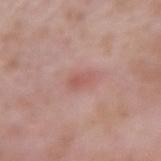Q: Is there a histopathology result?
A: catalogued during a skin exam; not biopsied
Q: Who is the patient?
A: male, about 55 years old
Q: What is the lesion's diameter?
A: about 2.5 mm
Q: Automated lesion metrics?
A: an area of roughly 3.5 mm² and a shape-asymmetry score of about 0.3 (0 = symmetric)
Q: What is the imaging modality?
A: ~15 mm tile from a whole-body skin photo
Q: Lesion location?
A: the right upper arm